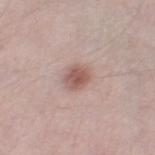| feature | finding |
|---|---|
| body site | the right thigh |
| image | ~15 mm tile from a whole-body skin photo |
| lesion size | ≈2.5 mm |
| TBP lesion metrics | about 12 CIELAB-L* units darker than the surrounding skin; a nevus-likeness score of about 90/100 |
| subject | male, in their mid- to late 40s |